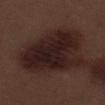Notes:
– workup · total-body-photography surveillance lesion; no biopsy
– illumination · white-light illumination
– imaging modality · ~15 mm tile from a whole-body skin photo
– site · the left thigh
– patient · male, about 70 years old